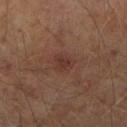The lesion was photographed on a routine skin check and not biopsied; there is no pathology result. Approximately 2.5 mm at its widest. A 15 mm crop from a total-body photograph taken for skin-cancer surveillance. The lesion is on the right lower leg. A male subject aged 63–67. The tile uses cross-polarized illumination.